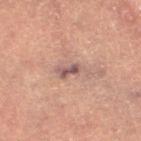notes = imaged on a skin check; not biopsied | automated lesion analysis = an area of roughly 5 mm² and a shape eccentricity near 0.85; a mean CIELAB color near L≈46 a*≈17 b*≈19 and roughly 9 lightness units darker than nearby skin; lesion-presence confidence of about 90/100 | patient = female, approximately 70 years of age | tile lighting = cross-polarized illumination | acquisition = ~15 mm tile from a whole-body skin photo | body site = the left thigh | diameter = about 3.5 mm.